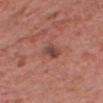Notes:
* notes · imaged on a skin check; not biopsied
* automated lesion analysis · a shape eccentricity near 0.8 and a shape-asymmetry score of about 0.2 (0 = symmetric); border irregularity of about 2 on a 0–10 scale and a within-lesion color-variation index near 2.5/10; a classifier nevus-likeness of about 20/100 and a detector confidence of about 100 out of 100 that the crop contains a lesion
* diameter · about 2.5 mm
* location · the head or neck
* patient · male, approximately 60 years of age
* illumination · white-light
* acquisition · 15 mm crop, total-body photography